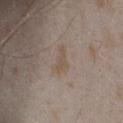Q: Is there a histopathology result?
A: total-body-photography surveillance lesion; no biopsy
Q: Lesion location?
A: the head or neck
Q: How was the tile lit?
A: white-light
Q: What is the lesion's diameter?
A: about 3.5 mm
Q: What is the imaging modality?
A: 15 mm crop, total-body photography
Q: Patient demographics?
A: female, about 25 years old